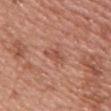No biopsy was performed on this lesion — it was imaged during a full skin examination and was not determined to be concerning. The lesion's longest dimension is about 3.5 mm. Captured under white-light illumination. An algorithmic analysis of the crop reported a lesion color around L≈52 a*≈25 b*≈30 in CIELAB, about 7 CIELAB-L* units darker than the surrounding skin, and a lesion-to-skin contrast of about 5.5 (normalized; higher = more distinct). It also reported a border-irregularity rating of about 4/10, a color-variation rating of about 2/10, and a peripheral color-asymmetry measure near 0.5. And it measured a nevus-likeness score of about 0/100 and a detector confidence of about 100 out of 100 that the crop contains a lesion. Cropped from a total-body skin-imaging series; the visible field is about 15 mm. A female subject, aged 48 to 52. Located on the upper back.